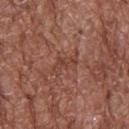Assessment:
This lesion was catalogued during total-body skin photography and was not selected for biopsy.